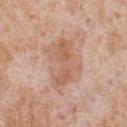Clinical impression:
The lesion was photographed on a routine skin check and not biopsied; there is no pathology result.
Clinical summary:
A 15 mm close-up extracted from a 3D total-body photography capture. The patient is a male approximately 65 years of age. The tile uses white-light illumination. The lesion is located on the chest. Measured at roughly 5.5 mm in maximum diameter. An algorithmic analysis of the crop reported a mean CIELAB color near L≈59 a*≈21 b*≈32 and roughly 9 lightness units darker than nearby skin. The software also gave a border-irregularity rating of about 5.5/10 and internal color variation of about 3 on a 0–10 scale. It also reported a nevus-likeness score of about 0/100 and a lesion-detection confidence of about 100/100.